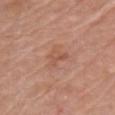Clinical impression: Part of a total-body skin-imaging series; this lesion was reviewed on a skin check and was not flagged for biopsy. Acquisition and patient details: The lesion is located on the chest. Cropped from a whole-body photographic skin survey; the tile spans about 15 mm. Imaged with white-light lighting. The subject is a male about 80 years old. Approximately 2.5 mm at its widest.